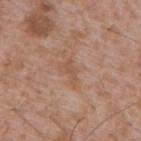| feature | finding |
|---|---|
| workup | imaged on a skin check; not biopsied |
| image source | ~15 mm crop, total-body skin-cancer survey |
| subject | male, roughly 45 years of age |
| automated metrics | a classifier nevus-likeness of about 0/100 and a lesion-detection confidence of about 100/100 |
| lesion diameter | ≈3 mm |
| lighting | white-light illumination |
| body site | the upper back |